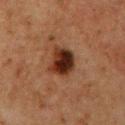Located on the back. A female patient, aged around 60. A roughly 15 mm field-of-view crop from a total-body skin photograph.